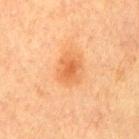Approximately 2.5 mm at its widest. This is a cross-polarized tile. From the left upper arm. A male patient aged 63 to 67. A 15 mm close-up tile from a total-body photography series done for melanoma screening.